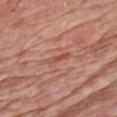A male subject aged approximately 60. The total-body-photography lesion software estimated a footprint of about 3 mm², a shape eccentricity near 0.95, and a shape-asymmetry score of about 0.4 (0 = symmetric). It also reported a classifier nevus-likeness of about 0/100. A lesion tile, about 15 mm wide, cut from a 3D total-body photograph. Captured under white-light illumination. On the chest. The recorded lesion diameter is about 3 mm.